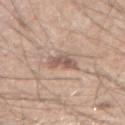Impression:
Recorded during total-body skin imaging; not selected for excision or biopsy.
Acquisition and patient details:
From the arm. A male subject in their mid- to late 40s. A lesion tile, about 15 mm wide, cut from a 3D total-body photograph.